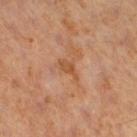The lesion was photographed on a routine skin check and not biopsied; there is no pathology result. About 3 mm across. From the leg. A 15 mm close-up extracted from a 3D total-body photography capture. This is a cross-polarized tile. A male subject, aged 58 to 62.